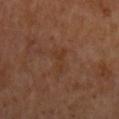  biopsy_status: not biopsied; imaged during a skin examination
  lighting: cross-polarized
  patient:
    sex: male
    age_approx: 65
  lesion_size:
    long_diameter_mm_approx: 3.5
  image:
    source: total-body photography crop
    field_of_view_mm: 15